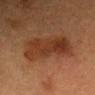Q: What did automated image analysis measure?
A: a footprint of about 20 mm², a shape eccentricity near 0.85, and a symmetry-axis asymmetry near 0.2; a lesion-detection confidence of about 100/100
Q: What is the anatomic site?
A: the head or neck
Q: What kind of image is this?
A: ~15 mm tile from a whole-body skin photo
Q: Lesion size?
A: ≈7 mm
Q: What lighting was used for the tile?
A: cross-polarized illumination
Q: Patient demographics?
A: female, in their 40s